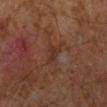workup: catalogued during a skin exam; not biopsied
site: the left lower leg
lesion size: ~3 mm (longest diameter)
illumination: cross-polarized illumination
subject: male, about 60 years old
image source: ~15 mm tile from a whole-body skin photo